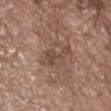Clinical impression:
No biopsy was performed on this lesion — it was imaged during a full skin examination and was not determined to be concerning.
Clinical summary:
A male patient, aged around 80. The tile uses white-light illumination. Cropped from a whole-body photographic skin survey; the tile spans about 15 mm. On the abdomen. An algorithmic analysis of the crop reported an average lesion color of about L≈46 a*≈18 b*≈25 (CIELAB) and about 8 CIELAB-L* units darker than the surrounding skin. And it measured a border-irregularity rating of about 7.5/10 and radial color variation of about 0.5. The software also gave a nevus-likeness score of about 5/100 and a detector confidence of about 100 out of 100 that the crop contains a lesion.